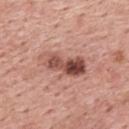The lesion was photographed on a routine skin check and not biopsied; there is no pathology result. The lesion is on the upper back. The tile uses white-light illumination. Approximately 6 mm at its widest. A 15 mm close-up tile from a total-body photography series done for melanoma screening. A male patient, aged 58 to 62.